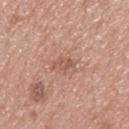Recorded during total-body skin imaging; not selected for excision or biopsy.
A roughly 15 mm field-of-view crop from a total-body skin photograph.
On the upper back.
The subject is a male in their 40s.